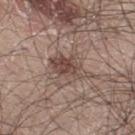follow-up — total-body-photography surveillance lesion; no biopsy | site — the right thigh | tile lighting — white-light illumination | image-analysis metrics — a shape eccentricity near 0.7 and a shape-asymmetry score of about 0.4 (0 = symmetric); a lesion–skin lightness drop of about 9 and a lesion-to-skin contrast of about 7 (normalized; higher = more distinct) | subject — male, in their 30s | image — ~15 mm crop, total-body skin-cancer survey.